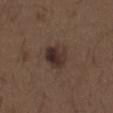Q: Is there a histopathology result?
A: imaged on a skin check; not biopsied
Q: Patient demographics?
A: male, approximately 50 years of age
Q: Illumination type?
A: white-light
Q: What is the imaging modality?
A: total-body-photography crop, ~15 mm field of view
Q: Where on the body is the lesion?
A: the abdomen
Q: Lesion size?
A: ≈3.5 mm